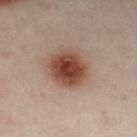Q: Is there a histopathology result?
A: no biopsy performed (imaged during a skin exam)
Q: What is the lesion's diameter?
A: ~4.5 mm (longest diameter)
Q: What is the anatomic site?
A: the left leg
Q: What lighting was used for the tile?
A: cross-polarized illumination
Q: Patient demographics?
A: male, in their 50s
Q: How was this image acquired?
A: total-body-photography crop, ~15 mm field of view